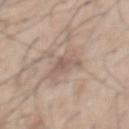notes: total-body-photography surveillance lesion; no biopsy
size: ~3 mm (longest diameter)
image-analysis metrics: a footprint of about 4 mm², an outline eccentricity of about 0.7 (0 = round, 1 = elongated), and a symmetry-axis asymmetry near 0.55; roughly 8 lightness units darker than nearby skin; lesion-presence confidence of about 55/100
image source: total-body-photography crop, ~15 mm field of view
body site: the chest
patient: male, aged 58 to 62